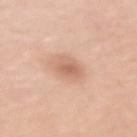Findings:
• follow-up: catalogued during a skin exam; not biopsied
• acquisition: ~15 mm tile from a whole-body skin photo
• lesion diameter: about 3.5 mm
• patient: female, aged 63–67
• body site: the left upper arm
• illumination: white-light